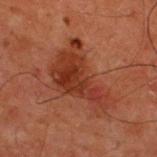A 15 mm close-up tile from a total-body photography series done for melanoma screening. The subject is a male aged 48 to 52. Captured under cross-polarized illumination. The lesion is on the upper back.